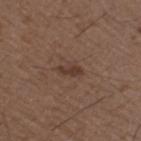Impression: Recorded during total-body skin imaging; not selected for excision or biopsy. Background: A lesion tile, about 15 mm wide, cut from a 3D total-body photograph. A male patient aged around 50. On the right upper arm. Measured at roughly 2.5 mm in maximum diameter. The tile uses white-light illumination.